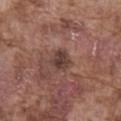<lesion>
<biopsy_status>not biopsied; imaged during a skin examination</biopsy_status>
</lesion>